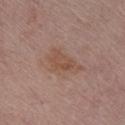  lighting: white-light
  image:
    source: total-body photography crop
    field_of_view_mm: 15
  patient:
    sex: female
    age_approx: 65
  automated_metrics:
    cielab_L: 50
    cielab_a: 19
    cielab_b: 27
    vs_skin_darker_L: 7.0
    vs_skin_contrast_norm: 6.0
  site: front of the torso
  lesion_size:
    long_diameter_mm_approx: 4.5